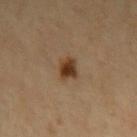Notes:
• biopsy status — catalogued during a skin exam; not biopsied
• lighting — cross-polarized illumination
• patient — male, aged approximately 65
• diameter — about 2.5 mm
• anatomic site — the left upper arm
• imaging modality — ~15 mm tile from a whole-body skin photo
• automated metrics — a lesion area of about 4.5 mm², an outline eccentricity of about 0.55 (0 = round, 1 = elongated), and a shape-asymmetry score of about 0.2 (0 = symmetric); an average lesion color of about L≈37 a*≈18 b*≈31 (CIELAB), roughly 13 lightness units darker than nearby skin, and a lesion-to-skin contrast of about 11.5 (normalized; higher = more distinct); a border-irregularity index near 2/10, a color-variation rating of about 4.5/10, and a peripheral color-asymmetry measure near 1.5; a classifier nevus-likeness of about 100/100 and a detector confidence of about 100 out of 100 that the crop contains a lesion